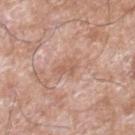Captured during whole-body skin photography for melanoma surveillance; the lesion was not biopsied.